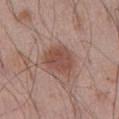{
  "biopsy_status": "not biopsied; imaged during a skin examination",
  "site": "abdomen",
  "lighting": "white-light",
  "image": {
    "source": "total-body photography crop",
    "field_of_view_mm": 15
  },
  "lesion_size": {
    "long_diameter_mm_approx": 4.0
  },
  "patient": {
    "sex": "male",
    "age_approx": 55
  },
  "automated_metrics": {
    "eccentricity": 0.45,
    "shape_asymmetry": 0.2,
    "cielab_L": 49,
    "cielab_a": 21,
    "cielab_b": 25,
    "vs_skin_contrast_norm": 7.5,
    "nevus_likeness_0_100": 90,
    "lesion_detection_confidence_0_100": 100
  }
}